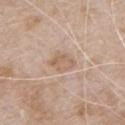Part of a total-body skin-imaging series; this lesion was reviewed on a skin check and was not flagged for biopsy.
This image is a 15 mm lesion crop taken from a total-body photograph.
A male subject, in their 80s.
The recorded lesion diameter is about 3.5 mm.
The lesion is on the chest.
Automated image analysis of the tile measured an eccentricity of roughly 0.7 and a symmetry-axis asymmetry near 0.25. It also reported a border-irregularity rating of about 2.5/10 and internal color variation of about 3 on a 0–10 scale. And it measured a nevus-likeness score of about 0/100 and a lesion-detection confidence of about 100/100.